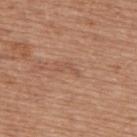No biopsy was performed on this lesion — it was imaged during a full skin examination and was not determined to be concerning. Located on the upper back. Cropped from a total-body skin-imaging series; the visible field is about 15 mm. A male patient, aged around 65. The recorded lesion diameter is about 2.5 mm. The tile uses white-light illumination.